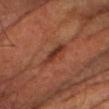workup: total-body-photography surveillance lesion; no biopsy
lighting: cross-polarized
automated metrics: border irregularity of about 3 on a 0–10 scale, a color-variation rating of about 3.5/10, and peripheral color asymmetry of about 1; an automated nevus-likeness rating near 0 out of 100 and lesion-presence confidence of about 100/100
site: the head or neck
subject: male, aged 63 to 67
lesion diameter: ~4 mm (longest diameter)
image source: 15 mm crop, total-body photography